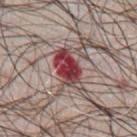The patient is a male aged 68 to 72.
Longest diameter approximately 5.5 mm.
A roughly 15 mm field-of-view crop from a total-body skin photograph.
Located on the chest.